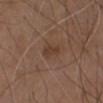Background: Automated image analysis of the tile measured a footprint of about 3.5 mm², a shape eccentricity near 0.75, and a symmetry-axis asymmetry near 0.25. And it measured a mean CIELAB color near L≈38 a*≈17 b*≈27, a lesion–skin lightness drop of about 6, and a normalized border contrast of about 6. And it measured a border-irregularity rating of about 2/10 and a within-lesion color-variation index near 1.5/10. The tile uses white-light illumination. A close-up tile cropped from a whole-body skin photograph, about 15 mm across. About 2.5 mm across. The lesion is on the chest. A male subject, in their mid- to late 70s.